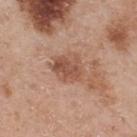- notes — total-body-photography surveillance lesion; no biopsy
- image — ~15 mm crop, total-body skin-cancer survey
- subject — male, roughly 55 years of age
- location — the upper back